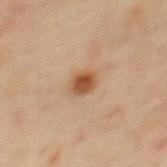Part of a total-body skin-imaging series; this lesion was reviewed on a skin check and was not flagged for biopsy. A 15 mm close-up tile from a total-body photography series done for melanoma screening. The patient is a male aged 43 to 47. Approximately 2.5 mm at its widest. The lesion is on the mid back. Captured under cross-polarized illumination.